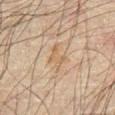Clinical impression:
Recorded during total-body skin imaging; not selected for excision or biopsy.
Context:
A roughly 15 mm field-of-view crop from a total-body skin photograph. The subject is a male about 70 years old. From the abdomen.